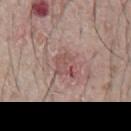<lesion>
  <biopsy_status>not biopsied; imaged during a skin examination</biopsy_status>
</lesion>